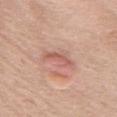Q: Is there a histopathology result?
A: no biopsy performed (imaged during a skin exam)
Q: Where on the body is the lesion?
A: the chest
Q: What kind of image is this?
A: ~15 mm crop, total-body skin-cancer survey
Q: What are the patient's age and sex?
A: female, in their mid-70s